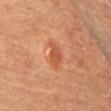This lesion was catalogued during total-body skin photography and was not selected for biopsy. A female patient, roughly 70 years of age. The lesion is on the chest. The lesion's longest dimension is about 3 mm. This image is a 15 mm lesion crop taken from a total-body photograph. This is a cross-polarized tile.